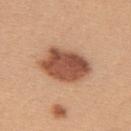This lesion was catalogued during total-body skin photography and was not selected for biopsy. The recorded lesion diameter is about 6 mm. The lesion is on the back. This image is a 15 mm lesion crop taken from a total-body photograph. Captured under white-light illumination. A female subject aged approximately 25.